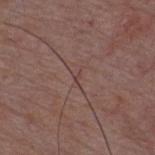biopsy status — total-body-photography surveillance lesion; no biopsy
lesion diameter — ≈1 mm
automated metrics — an area of roughly 0.5 mm², a shape eccentricity near 0.85, and a shape-asymmetry score of about 0.35 (0 = symmetric); border irregularity of about 3.5 on a 0–10 scale and a color-variation rating of about 0/10
lighting — white-light illumination
patient — male, roughly 50 years of age
site — the front of the torso
acquisition — ~15 mm tile from a whole-body skin photo